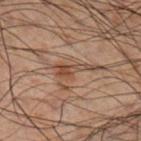workup = total-body-photography surveillance lesion; no biopsy | subject = male, in their 50s | acquisition = ~15 mm crop, total-body skin-cancer survey | anatomic site = the right lower leg | lesion size = about 4 mm | TBP lesion metrics = an average lesion color of about L≈35 a*≈15 b*≈23 (CIELAB), about 7 CIELAB-L* units darker than the surrounding skin, and a normalized border contrast of about 7; an automated nevus-likeness rating near 5 out of 100 | illumination = cross-polarized illumination.